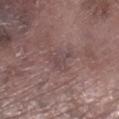Imaged during a routine full-body skin examination; the lesion was not biopsied and no histopathology is available. The tile uses white-light illumination. The lesion-visualizer software estimated an automated nevus-likeness rating near 0 out of 100. From the right lower leg. The recorded lesion diameter is about 3.5 mm. A male subject aged around 75. A 15 mm crop from a total-body photograph taken for skin-cancer surveillance.